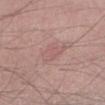Notes:
– workup: total-body-photography surveillance lesion; no biopsy
– anatomic site: the right forearm
– imaging modality: 15 mm crop, total-body photography
– subject: male, aged 48 to 52